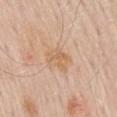| field | value |
|---|---|
| notes | catalogued during a skin exam; not biopsied |
| acquisition | ~15 mm tile from a whole-body skin photo |
| location | the mid back |
| tile lighting | white-light illumination |
| subject | male, aged 58 to 62 |